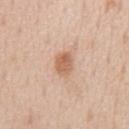No biopsy was performed on this lesion — it was imaged during a full skin examination and was not determined to be concerning. Located on the chest. Measured at roughly 3.5 mm in maximum diameter. Automated image analysis of the tile measured a shape eccentricity near 0.8 and a symmetry-axis asymmetry near 0.25. The software also gave a within-lesion color-variation index near 3.5/10 and radial color variation of about 1.5. A roughly 15 mm field-of-view crop from a total-body skin photograph. This is a white-light tile. A male subject, aged 53–57.